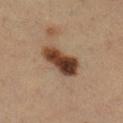| feature | finding |
|---|---|
| workup | total-body-photography surveillance lesion; no biopsy |
| image | total-body-photography crop, ~15 mm field of view |
| subject | female, aged around 30 |
| diameter | ~5.5 mm (longest diameter) |
| anatomic site | the leg |